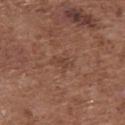Impression:
The lesion was photographed on a routine skin check and not biopsied; there is no pathology result.
Acquisition and patient details:
A lesion tile, about 15 mm wide, cut from a 3D total-body photograph. A female subject aged 73 to 77. Located on the upper back. Imaged with white-light lighting. The lesion's longest dimension is about 2.5 mm.